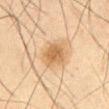{
  "biopsy_status": "not biopsied; imaged during a skin examination",
  "lighting": "cross-polarized",
  "automated_metrics": {
    "area_mm2_approx": 9.0,
    "eccentricity": 0.65,
    "shape_asymmetry": 0.15,
    "color_variation_0_10": 3.0,
    "peripheral_color_asymmetry": 1.0,
    "nevus_likeness_0_100": 75,
    "lesion_detection_confidence_0_100": 100
  },
  "lesion_size": {
    "long_diameter_mm_approx": 4.0
  },
  "site": "abdomen",
  "image": {
    "source": "total-body photography crop",
    "field_of_view_mm": 15
  },
  "patient": {
    "sex": "male",
    "age_approx": 60
  }
}